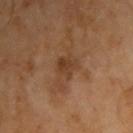Clinical impression: Recorded during total-body skin imaging; not selected for excision or biopsy. Acquisition and patient details: A 15 mm close-up extracted from a 3D total-body photography capture. Automated tile analysis of the lesion measured an automated nevus-likeness rating near 0 out of 100. A male patient, aged 58 to 62. Captured under cross-polarized illumination. Measured at roughly 2.5 mm in maximum diameter. From the right arm.